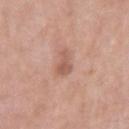The lesion was photographed on a routine skin check and not biopsied; there is no pathology result. The tile uses white-light illumination. A close-up tile cropped from a whole-body skin photograph, about 15 mm across. The subject is a male roughly 55 years of age. Located on the right upper arm. The total-body-photography lesion software estimated a lesion color around L≈57 a*≈22 b*≈28 in CIELAB and a normalized lesion–skin contrast near 6.5.